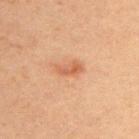Background:
This image is a 15 mm lesion crop taken from a total-body photograph. Approximately 3 mm at its widest. Automated image analysis of the tile measured a lesion color around L≈49 a*≈22 b*≈32 in CIELAB, roughly 8 lightness units darker than nearby skin, and a normalized border contrast of about 6.5. The analysis additionally found a border-irregularity rating of about 3/10, a within-lesion color-variation index near 3/10, and peripheral color asymmetry of about 1. Imaged with cross-polarized lighting. The patient is a male in their 30s. The lesion is on the upper back.